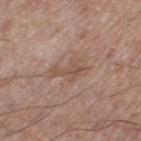Clinical impression: The lesion was tiled from a total-body skin photograph and was not biopsied. Context: A roughly 15 mm field-of-view crop from a total-body skin photograph. Captured under white-light illumination. The subject is a male aged approximately 50. The lesion is located on the right thigh. About 4 mm across.